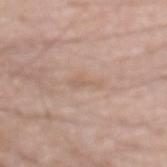Notes:
- follow-up · imaged on a skin check; not biopsied
- image · ~15 mm crop, total-body skin-cancer survey
- subject · male, roughly 55 years of age
- body site · the left forearm